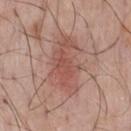Background: A male patient in their mid-40s. Imaged with white-light lighting. A 15 mm close-up tile from a total-body photography series done for melanoma screening. On the front of the torso. The lesion's longest dimension is about 6.5 mm.